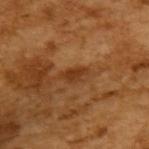Recorded during total-body skin imaging; not selected for excision or biopsy.
A male patient aged approximately 65.
This image is a 15 mm lesion crop taken from a total-body photograph.